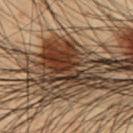No biopsy was performed on this lesion — it was imaged during a full skin examination and was not determined to be concerning. The subject is a male aged 53 to 57. The lesion-visualizer software estimated an outline eccentricity of about 0.8 (0 = round, 1 = elongated) and a shape-asymmetry score of about 0.55 (0 = symmetric). The software also gave a normalized border contrast of about 11. It also reported a border-irregularity index near 8/10, a color-variation rating of about 7.5/10, and peripheral color asymmetry of about 2.5. From the abdomen. A 15 mm crop from a total-body photograph taken for skin-cancer surveillance. Approximately 7 mm at its widest.